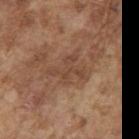Part of a total-body skin-imaging series; this lesion was reviewed on a skin check and was not flagged for biopsy. The lesion-visualizer software estimated an area of roughly 9 mm², an outline eccentricity of about 0.65 (0 = round, 1 = elongated), and a shape-asymmetry score of about 0.4 (0 = symmetric). And it measured a border-irregularity rating of about 6.5/10, a color-variation rating of about 2.5/10, and a peripheral color-asymmetry measure near 1. The recorded lesion diameter is about 4 mm. The lesion is located on the right upper arm. Imaged with white-light lighting. A region of skin cropped from a whole-body photographic capture, roughly 15 mm wide. A male subject, aged around 75.